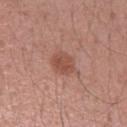Notes:
– follow-up: imaged on a skin check; not biopsied
– diameter: about 3 mm
– lighting: white-light
– patient: female, roughly 35 years of age
– acquisition: ~15 mm crop, total-body skin-cancer survey
– site: the right upper arm